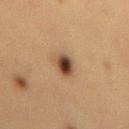This is a cross-polarized tile.
An algorithmic analysis of the crop reported a footprint of about 5.5 mm² and two-axis asymmetry of about 0.25. It also reported an average lesion color of about L≈37 a*≈16 b*≈27 (CIELAB), roughly 14 lightness units darker than nearby skin, and a normalized lesion–skin contrast near 11.5.
A lesion tile, about 15 mm wide, cut from a 3D total-body photograph.
A female subject aged 38–42.
From the back.